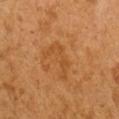The lesion was photographed on a routine skin check and not biopsied; there is no pathology result. Approximately 4.5 mm at its widest. Imaged with cross-polarized lighting. A region of skin cropped from a whole-body photographic capture, roughly 15 mm wide. A male patient, roughly 50 years of age. From the right upper arm.